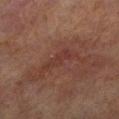biopsy status = imaged on a skin check; not biopsied